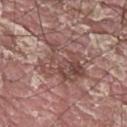follow-up = total-body-photography surveillance lesion; no biopsy
image = total-body-photography crop, ~15 mm field of view
size = about 6 mm
location = the upper back
patient = male, aged approximately 40